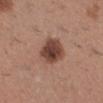  image:
    source: total-body photography crop
    field_of_view_mm: 15
  patient:
    sex: female
    age_approx: 30
  site: right lower leg